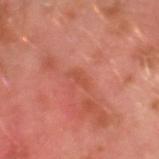No biopsy was performed on this lesion — it was imaged during a full skin examination and was not determined to be concerning. A male patient, about 30 years old. The lesion's longest dimension is about 2.5 mm. The tile uses cross-polarized illumination. A lesion tile, about 15 mm wide, cut from a 3D total-body photograph. The lesion is located on the left arm.